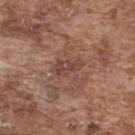Captured during whole-body skin photography for melanoma surveillance; the lesion was not biopsied. The patient is a male in their mid-70s. The lesion is on the back. Approximately 3.5 mm at its widest. Captured under white-light illumination. A lesion tile, about 15 mm wide, cut from a 3D total-body photograph.